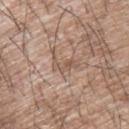Assessment: The lesion was photographed on a routine skin check and not biopsied; there is no pathology result. Image and clinical context: A male subject aged around 65. A 15 mm close-up tile from a total-body photography series done for melanoma screening. Imaged with white-light lighting. On the upper back.